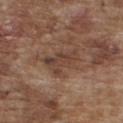The lesion was tiled from a total-body skin photograph and was not biopsied.
The lesion's longest dimension is about 4.5 mm.
Cropped from a total-body skin-imaging series; the visible field is about 15 mm.
The lesion is located on the chest.
A male patient, approximately 75 years of age.
An algorithmic analysis of the crop reported an average lesion color of about L≈41 a*≈18 b*≈26 (CIELAB), a lesion–skin lightness drop of about 8, and a normalized border contrast of about 7. The software also gave a color-variation rating of about 3/10 and a peripheral color-asymmetry measure near 1.
The tile uses white-light illumination.